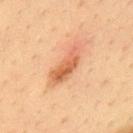The lesion was photographed on a routine skin check and not biopsied; there is no pathology result. A 15 mm close-up tile from a total-body photography series done for melanoma screening. From the upper back. A male patient about 35 years old.